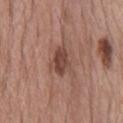The lesion was photographed on a routine skin check and not biopsied; there is no pathology result. About 4 mm across. Automated image analysis of the tile measured a lesion area of about 8 mm², a shape eccentricity near 0.75, and two-axis asymmetry of about 0.25. And it measured a lesion color around L≈45 a*≈21 b*≈25 in CIELAB and about 11 CIELAB-L* units darker than the surrounding skin. It also reported a border-irregularity index near 3/10. This is a white-light tile. Located on the chest. This image is a 15 mm lesion crop taken from a total-body photograph. A male patient, in their mid-50s.